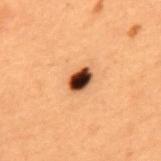The lesion was photographed on a routine skin check and not biopsied; there is no pathology result.
This is a cross-polarized tile.
A 15 mm close-up tile from a total-body photography series done for melanoma screening.
Approximately 3 mm at its widest.
The lesion is located on the mid back.
The subject is a male roughly 50 years of age.